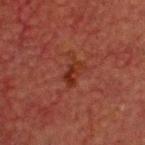{"biopsy_status": "not biopsied; imaged during a skin examination", "lighting": "cross-polarized", "site": "head or neck", "image": {"source": "total-body photography crop", "field_of_view_mm": 15}, "patient": {"sex": "male", "age_approx": 60}}